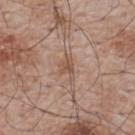The lesion was photographed on a routine skin check and not biopsied; there is no pathology result.
Cropped from a whole-body photographic skin survey; the tile spans about 15 mm.
The subject is a male aged 63–67.
Approximately 2.5 mm at its widest.
Captured under white-light illumination.
The lesion is located on the upper back.